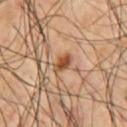<case>
  <biopsy_status>not biopsied; imaged during a skin examination</biopsy_status>
  <patient>
    <sex>male</sex>
    <age_approx>60</age_approx>
  </patient>
  <site>chest</site>
  <image>
    <source>total-body photography crop</source>
    <field_of_view_mm>15</field_of_view_mm>
  </image>
  <lighting>cross-polarized</lighting>
  <lesion_size>
    <long_diameter_mm_approx>3.0</long_diameter_mm_approx>
  </lesion_size>
</case>